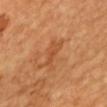follow-up=imaged on a skin check; not biopsied | tile lighting=cross-polarized illumination | anatomic site=the mid back | image-analysis metrics=a footprint of about 6 mm² and an eccentricity of roughly 0.85 | acquisition=15 mm crop, total-body photography | patient=male, aged 63–67.